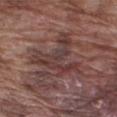<case>
  <biopsy_status>not biopsied; imaged during a skin examination</biopsy_status>
  <automated_metrics>
    <cielab_L>38</cielab_L>
    <cielab_a>19</cielab_a>
    <cielab_b>20</cielab_b>
    <vs_skin_contrast_norm>7.0</vs_skin_contrast_norm>
    <border_irregularity_0_10>10.0</border_irregularity_0_10>
    <peripheral_color_asymmetry>2.0</peripheral_color_asymmetry>
  </automated_metrics>
  <image>
    <source>total-body photography crop</source>
    <field_of_view_mm>15</field_of_view_mm>
  </image>
  <lesion_size>
    <long_diameter_mm_approx>6.5</long_diameter_mm_approx>
  </lesion_size>
  <site>right upper arm</site>
  <patient>
    <sex>male</sex>
    <age_approx>75</age_approx>
  </patient>
  <lighting>white-light</lighting>
</case>